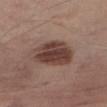| key | value |
|---|---|
| lesion diameter | ≈6 mm |
| subject | male, roughly 75 years of age |
| anatomic site | the leg |
| image source | total-body-photography crop, ~15 mm field of view |